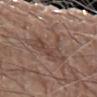Q: Was this lesion biopsied?
A: imaged on a skin check; not biopsied
Q: How was this image acquired?
A: ~15 mm tile from a whole-body skin photo
Q: Patient demographics?
A: male, in their 80s
Q: Where on the body is the lesion?
A: the arm
Q: What lighting was used for the tile?
A: white-light
Q: What is the lesion's diameter?
A: ≈6 mm
Q: Automated lesion metrics?
A: a footprint of about 16 mm², a shape eccentricity near 0.8, and a symmetry-axis asymmetry near 0.5; a lesion color around L≈45 a*≈17 b*≈24 in CIELAB, roughly 7 lightness units darker than nearby skin, and a lesion-to-skin contrast of about 5.5 (normalized; higher = more distinct); border irregularity of about 8 on a 0–10 scale, a color-variation rating of about 3.5/10, and radial color variation of about 1